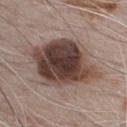biopsy status — total-body-photography surveillance lesion; no biopsy | automated metrics — a border-irregularity index near 2.5/10, a color-variation rating of about 7.5/10, and a peripheral color-asymmetry measure near 2; a classifier nevus-likeness of about 65/100 and lesion-presence confidence of about 100/100 | tile lighting — white-light | body site — the chest | acquisition — 15 mm crop, total-body photography | patient — male, aged 68–72 | lesion size — about 9 mm.